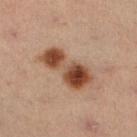No biopsy was performed on this lesion — it was imaged during a full skin examination and was not determined to be concerning. The total-body-photography lesion software estimated a lesion area of about 16 mm², an eccentricity of roughly 0.9, and two-axis asymmetry of about 0.3. And it measured an average lesion color of about L≈46 a*≈21 b*≈31 (CIELAB), a lesion–skin lightness drop of about 15, and a normalized border contrast of about 11. The analysis additionally found a border-irregularity index near 4/10, a color-variation rating of about 10/10, and a peripheral color-asymmetry measure near 3.5. The analysis additionally found an automated nevus-likeness rating near 100 out of 100 and a lesion-detection confidence of about 100/100. From the right lower leg. A lesion tile, about 15 mm wide, cut from a 3D total-body photograph. About 6.5 mm across. A male subject, aged around 55. This is a cross-polarized tile.